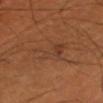| key | value |
|---|---|
| follow-up | catalogued during a skin exam; not biopsied |
| automated lesion analysis | a lesion area of about 11 mm², an outline eccentricity of about 0.8 (0 = round, 1 = elongated), and a shape-asymmetry score of about 0.55 (0 = symmetric); an average lesion color of about L≈39 a*≈21 b*≈31 (CIELAB), a lesion–skin lightness drop of about 5, and a normalized border contrast of about 4.5 |
| acquisition | ~15 mm crop, total-body skin-cancer survey |
| site | the right thigh |
| lesion size | about 5.5 mm |
| patient | male, approximately 50 years of age |